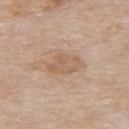workup: total-body-photography surveillance lesion; no biopsy
lighting: white-light
site: the upper back
automated lesion analysis: a lesion area of about 8 mm², an outline eccentricity of about 0.8 (0 = round, 1 = elongated), and a symmetry-axis asymmetry near 0.25; border irregularity of about 2.5 on a 0–10 scale, a color-variation rating of about 3/10, and peripheral color asymmetry of about 1
imaging modality: ~15 mm crop, total-body skin-cancer survey
patient: male, aged approximately 85 or older